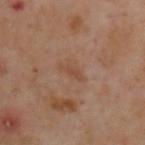The lesion was photographed on a routine skin check and not biopsied; there is no pathology result. A 15 mm crop from a total-body photograph taken for skin-cancer surveillance. From the upper back. Measured at roughly 3 mm in maximum diameter. A male patient approximately 55 years of age.